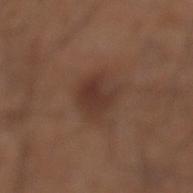* acquisition — total-body-photography crop, ~15 mm field of view
* subject — male, roughly 60 years of age
* anatomic site — the left lower leg